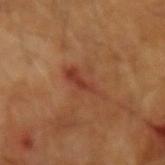| field | value |
|---|---|
| follow-up | imaged on a skin check; not biopsied |
| subject | male, in their mid- to late 60s |
| acquisition | ~15 mm tile from a whole-body skin photo |